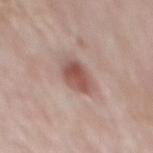Imaged during a routine full-body skin examination; the lesion was not biopsied and no histopathology is available.
Located on the mid back.
A 15 mm close-up tile from a total-body photography series done for melanoma screening.
A male patient in their mid- to late 50s.